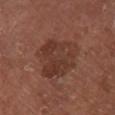Captured during whole-body skin photography for melanoma surveillance; the lesion was not biopsied. A male patient, in their mid-60s. This image is a 15 mm lesion crop taken from a total-body photograph. Approximately 5.5 mm at its widest. The lesion is on the right lower leg.